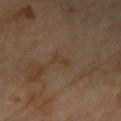<tbp_lesion>
  <biopsy_status>not biopsied; imaged during a skin examination</biopsy_status>
  <patient>
    <sex>female</sex>
    <age_approx>70</age_approx>
  </patient>
  <site>right forearm</site>
  <lesion_size>
    <long_diameter_mm_approx>2.5</long_diameter_mm_approx>
  </lesion_size>
  <image>
    <source>total-body photography crop</source>
    <field_of_view_mm>15</field_of_view_mm>
  </image>
  <automated_metrics>
    <eccentricity>0.85</eccentricity>
    <shape_asymmetry>0.4</shape_asymmetry>
    <nevus_likeness_0_100>0</nevus_likeness_0_100>
    <lesion_detection_confidence_0_100>100</lesion_detection_confidence_0_100>
  </automated_metrics>
  <lighting>cross-polarized</lighting>
</tbp_lesion>